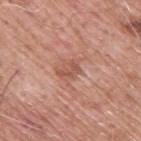Located on the upper back. About 2.5 mm across. A 15 mm crop from a total-body photograph taken for skin-cancer surveillance. This is a white-light tile. A male subject, about 60 years old.